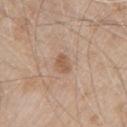The lesion was photographed on a routine skin check and not biopsied; there is no pathology result. A male subject aged approximately 80. The lesion's longest dimension is about 2.5 mm. From the chest. This is a white-light tile. A 15 mm crop from a total-body photograph taken for skin-cancer surveillance. An algorithmic analysis of the crop reported a lesion area of about 3.5 mm², an outline eccentricity of about 0.75 (0 = round, 1 = elongated), and a symmetry-axis asymmetry near 0.25. And it measured a lesion–skin lightness drop of about 9 and a normalized border contrast of about 6.5. The analysis additionally found a border-irregularity index near 2/10, internal color variation of about 1.5 on a 0–10 scale, and radial color variation of about 0.5. The software also gave a nevus-likeness score of about 40/100 and lesion-presence confidence of about 100/100.